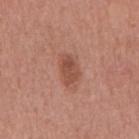The lesion was tiled from a total-body skin photograph and was not biopsied. The tile uses white-light illumination. A male patient, roughly 45 years of age. The lesion is on the chest. This image is a 15 mm lesion crop taken from a total-body photograph. Longest diameter approximately 4 mm.